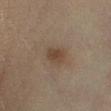The lesion was tiled from a total-body skin photograph and was not biopsied.
Captured under cross-polarized illumination.
The recorded lesion diameter is about 2.5 mm.
The lesion is on the left lower leg.
A female subject in their mid- to late 50s.
Cropped from a total-body skin-imaging series; the visible field is about 15 mm.